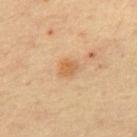Clinical impression:
Captured during whole-body skin photography for melanoma surveillance; the lesion was not biopsied.
Clinical summary:
The subject is a male approximately 65 years of age. The recorded lesion diameter is about 2.5 mm. From the front of the torso. A 15 mm crop from a total-body photograph taken for skin-cancer surveillance. This is a cross-polarized tile.